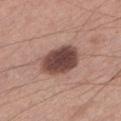The lesion was tiled from a total-body skin photograph and was not biopsied.
Located on the left lower leg.
A male subject aged approximately 50.
A 15 mm crop from a total-body photograph taken for skin-cancer surveillance.
Automated tile analysis of the lesion measured a footprint of about 15 mm², a shape eccentricity near 0.75, and a symmetry-axis asymmetry near 0.15. The software also gave border irregularity of about 1.5 on a 0–10 scale and internal color variation of about 4.5 on a 0–10 scale. It also reported lesion-presence confidence of about 100/100.
Imaged with white-light lighting.